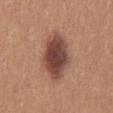Case summary:
- follow-up: total-body-photography surveillance lesion; no biopsy
- image: total-body-photography crop, ~15 mm field of view
- image-analysis metrics: a lesion area of about 18 mm², an outline eccentricity of about 0.85 (0 = round, 1 = elongated), and a shape-asymmetry score of about 0.15 (0 = symmetric); a border-irregularity index near 2/10, internal color variation of about 5.5 on a 0–10 scale, and radial color variation of about 1.5
- lighting: white-light
- anatomic site: the mid back
- patient: female, about 40 years old